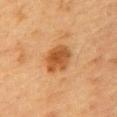| key | value |
|---|---|
| follow-up | imaged on a skin check; not biopsied |
| lighting | cross-polarized |
| subject | male, aged 83 or older |
| image | total-body-photography crop, ~15 mm field of view |
| automated metrics | an area of roughly 9.5 mm², a shape eccentricity near 0.6, and two-axis asymmetry of about 0.15; a lesion color around L≈47 a*≈23 b*≈38 in CIELAB, about 11 CIELAB-L* units darker than the surrounding skin, and a normalized lesion–skin contrast near 8.5; border irregularity of about 1.5 on a 0–10 scale and a color-variation rating of about 4/10 |
| location | the chest |